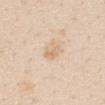<case>
  <biopsy_status>not biopsied; imaged during a skin examination</biopsy_status>
  <site>mid back</site>
  <patient>
    <sex>female</sex>
    <age_approx>45</age_approx>
  </patient>
  <image>
    <source>total-body photography crop</source>
    <field_of_view_mm>15</field_of_view_mm>
  </image>
</case>